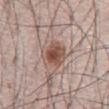Captured during whole-body skin photography for melanoma surveillance; the lesion was not biopsied.